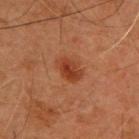Context: A 15 mm close-up tile from a total-body photography series done for melanoma screening. Measured at roughly 3.5 mm in maximum diameter. A male patient, in their mid-40s. Captured under cross-polarized illumination. On the back.